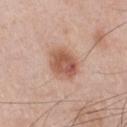• workup · total-body-photography surveillance lesion; no biopsy
• image · 15 mm crop, total-body photography
• automated metrics · about 12 CIELAB-L* units darker than the surrounding skin and a lesion-to-skin contrast of about 8.5 (normalized; higher = more distinct); a border-irregularity index near 1.5/10, a within-lesion color-variation index near 5.5/10, and peripheral color asymmetry of about 2
• subject · male, approximately 60 years of age
• body site · the chest